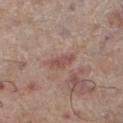{"biopsy_status": "not biopsied; imaged during a skin examination", "lesion_size": {"long_diameter_mm_approx": 3.5}, "lighting": "white-light", "image": {"source": "total-body photography crop", "field_of_view_mm": 15}, "patient": {"sex": "male", "age_approx": 70}, "automated_metrics": {"area_mm2_approx": 4.0, "eccentricity": 0.9, "shape_asymmetry": 0.35, "cielab_L": 50, "cielab_a": 21, "cielab_b": 23, "border_irregularity_0_10": 3.5, "nevus_likeness_0_100": 0, "lesion_detection_confidence_0_100": 90}, "site": "left lower leg"}